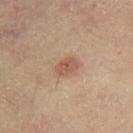This lesion was catalogued during total-body skin photography and was not selected for biopsy. The subject is a female aged around 45. Captured under cross-polarized illumination. The lesion is on the right thigh. A 15 mm crop from a total-body photograph taken for skin-cancer surveillance. About 2.5 mm across.